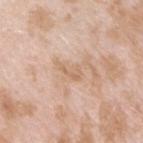Impression: No biopsy was performed on this lesion — it was imaged during a full skin examination and was not determined to be concerning. Background: On the right upper arm. This is a white-light tile. About 3 mm across. Automated image analysis of the tile measured a classifier nevus-likeness of about 0/100 and a detector confidence of about 100 out of 100 that the crop contains a lesion. A female patient about 25 years old. A 15 mm crop from a total-body photograph taken for skin-cancer surveillance.